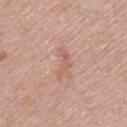A female patient approximately 65 years of age. The total-body-photography lesion software estimated an area of roughly 4.5 mm², an outline eccentricity of about 0.9 (0 = round, 1 = elongated), and a shape-asymmetry score of about 0.6 (0 = symmetric). And it measured a detector confidence of about 100 out of 100 that the crop contains a lesion. This is a white-light tile. Cropped from a whole-body photographic skin survey; the tile spans about 15 mm. About 4 mm across. The lesion is on the upper back.